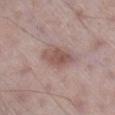<lesion>
  <biopsy_status>not biopsied; imaged during a skin examination</biopsy_status>
  <lesion_size>
    <long_diameter_mm_approx>4.0</long_diameter_mm_approx>
  </lesion_size>
  <site>right lower leg</site>
  <automated_metrics>
    <border_irregularity_0_10>2.5</border_irregularity_0_10>
    <color_variation_0_10>3.0</color_variation_0_10>
    <peripheral_color_asymmetry>1.0</peripheral_color_asymmetry>
  </automated_metrics>
  <patient>
    <sex>male</sex>
    <age_approx>60</age_approx>
  </patient>
  <image>
    <source>total-body photography crop</source>
    <field_of_view_mm>15</field_of_view_mm>
  </image>
</lesion>